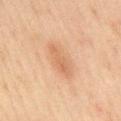Case summary:
– biopsy status — total-body-photography surveillance lesion; no biopsy
– lesion size — about 4.5 mm
– image — 15 mm crop, total-body photography
– location — the mid back
– subject — female, aged 58–62
– illumination — cross-polarized illumination
– automated lesion analysis — a lesion–skin lightness drop of about 7 and a normalized lesion–skin contrast near 5.5; a nevus-likeness score of about 40/100 and lesion-presence confidence of about 100/100